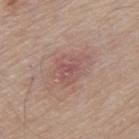{
  "biopsy_status": "not biopsied; imaged during a skin examination",
  "lighting": "white-light",
  "lesion_size": {
    "long_diameter_mm_approx": 4.5
  },
  "patient": {
    "sex": "male",
    "age_approx": 80
  },
  "image": {
    "source": "total-body photography crop",
    "field_of_view_mm": 15
  },
  "site": "mid back"
}